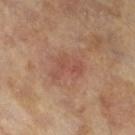The lesion was tiled from a total-body skin photograph and was not biopsied. The tile uses cross-polarized illumination. An algorithmic analysis of the crop reported a footprint of about 6.5 mm², an eccentricity of roughly 0.8, and a symmetry-axis asymmetry near 0.5. And it measured a lesion color around L≈48 a*≈22 b*≈27 in CIELAB, a lesion–skin lightness drop of about 7, and a normalized border contrast of about 5. And it measured a classifier nevus-likeness of about 5/100 and a detector confidence of about 100 out of 100 that the crop contains a lesion. A male patient aged approximately 65. Approximately 4 mm at its widest. A region of skin cropped from a whole-body photographic capture, roughly 15 mm wide.